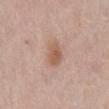Impression: No biopsy was performed on this lesion — it was imaged during a full skin examination and was not determined to be concerning. Context: A male patient, aged 73–77. Located on the front of the torso. A roughly 15 mm field-of-view crop from a total-body skin photograph. Measured at roughly 3 mm in maximum diameter. Automated tile analysis of the lesion measured a border-irregularity index near 2/10 and a within-lesion color-variation index near 3/10. And it measured a classifier nevus-likeness of about 80/100 and a lesion-detection confidence of about 100/100. Captured under white-light illumination.